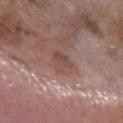Imaged during a routine full-body skin examination; the lesion was not biopsied and no histopathology is available. A lesion tile, about 15 mm wide, cut from a 3D total-body photograph. The lesion is on the left forearm. A male patient aged 58 to 62.